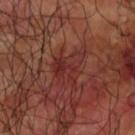Clinical impression:
The lesion was tiled from a total-body skin photograph and was not biopsied.
Context:
A male subject aged 58–62. On the right upper arm. A roughly 15 mm field-of-view crop from a total-body skin photograph. An algorithmic analysis of the crop reported a lesion area of about 8.5 mm², a shape eccentricity near 0.5, and two-axis asymmetry of about 0.45. It also reported a mean CIELAB color near L≈30 a*≈26 b*≈23 and about 5 CIELAB-L* units darker than the surrounding skin. It also reported a nevus-likeness score of about 0/100 and a detector confidence of about 90 out of 100 that the crop contains a lesion.